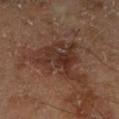Q: Illumination type?
A: cross-polarized
Q: What kind of image is this?
A: ~15 mm crop, total-body skin-cancer survey
Q: How large is the lesion?
A: about 7 mm
Q: What are the patient's age and sex?
A: male, about 70 years old
Q: What is the anatomic site?
A: the right lower leg